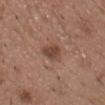Imaged during a routine full-body skin examination; the lesion was not biopsied and no histopathology is available.
The patient is a male in their mid- to late 50s.
A 15 mm close-up tile from a total-body photography series done for melanoma screening.
The tile uses white-light illumination.
On the front of the torso.